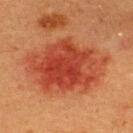Q: Was a biopsy performed?
A: imaged on a skin check; not biopsied
Q: Automated lesion metrics?
A: a lesion area of about 46 mm², an eccentricity of roughly 0.75, and a shape-asymmetry score of about 0.2 (0 = symmetric); a border-irregularity rating of about 3/10, internal color variation of about 5.5 on a 0–10 scale, and peripheral color asymmetry of about 1.5; an automated nevus-likeness rating near 100 out of 100
Q: What is the lesion's diameter?
A: about 10 mm
Q: What is the imaging modality?
A: 15 mm crop, total-body photography
Q: Who is the patient?
A: male, aged 38–42
Q: How was the tile lit?
A: cross-polarized illumination
Q: What is the anatomic site?
A: the back